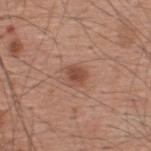| field | value |
|---|---|
| notes | imaged on a skin check; not biopsied |
| illumination | white-light |
| patient | male, aged approximately 60 |
| site | the upper back |
| acquisition | total-body-photography crop, ~15 mm field of view |
| lesion size | ≈2.5 mm |